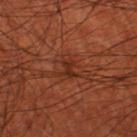Impression: This lesion was catalogued during total-body skin photography and was not selected for biopsy. Context: The lesion is located on the left thigh. Measured at roughly 3 mm in maximum diameter. A roughly 15 mm field-of-view crop from a total-body skin photograph. This is a cross-polarized tile. A male subject roughly 70 years of age.